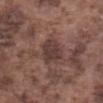lesion diameter: ~4 mm (longest diameter) | image: total-body-photography crop, ~15 mm field of view | subject: male, aged 73–77 | location: the abdomen | tile lighting: white-light illumination | automated metrics: a lesion area of about 9 mm², an outline eccentricity of about 0.7 (0 = round, 1 = elongated), and a shape-asymmetry score of about 0.25 (0 = symmetric); internal color variation of about 2 on a 0–10 scale and radial color variation of about 1.